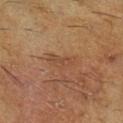A region of skin cropped from a whole-body photographic capture, roughly 15 mm wide.
The lesion is on the right lower leg.
A male patient approximately 60 years of age.